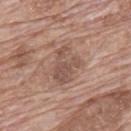{
  "biopsy_status": "not biopsied; imaged during a skin examination",
  "patient": {
    "sex": "male",
    "age_approx": 70
  },
  "image": {
    "source": "total-body photography crop",
    "field_of_view_mm": 15
  },
  "site": "upper back"
}